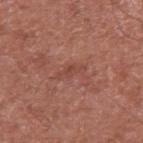  biopsy_status: not biopsied; imaged during a skin examination
  lighting: white-light
  automated_metrics:
    area_mm2_approx: 4.0
    eccentricity: 0.9
    shape_asymmetry: 0.35
    color_variation_0_10: 1.5
    peripheral_color_asymmetry: 0.5
    nevus_likeness_0_100: 0
    lesion_detection_confidence_0_100: 100
  lesion_size:
    long_diameter_mm_approx: 3.5
  patient:
    sex: male
    age_approx: 65
  site: arm
  image:
    source: total-body photography crop
    field_of_view_mm: 15